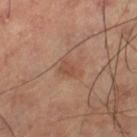| key | value |
|---|---|
| TBP lesion metrics | a footprint of about 3.5 mm², an eccentricity of roughly 0.75, and two-axis asymmetry of about 0.3; roughly 7 lightness units darker than nearby skin and a lesion-to-skin contrast of about 5.5 (normalized; higher = more distinct); a border-irregularity rating of about 3/10, a within-lesion color-variation index near 1.5/10, and radial color variation of about 0.5; an automated nevus-likeness rating near 15 out of 100 and a lesion-detection confidence of about 100/100 |
| lesion size | ≈2.5 mm |
| imaging modality | total-body-photography crop, ~15 mm field of view |
| lighting | cross-polarized illumination |
| subject | male, aged 58–62 |
| site | the left thigh |